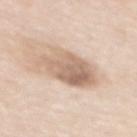Findings:
– follow-up · imaged on a skin check; not biopsied
– patient · female, aged 48–52
– TBP lesion metrics · a classifier nevus-likeness of about 40/100 and a detector confidence of about 100 out of 100 that the crop contains a lesion
– lesion size · ~6 mm (longest diameter)
– illumination · white-light
– acquisition · 15 mm crop, total-body photography
– location · the mid back